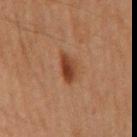The lesion was photographed on a routine skin check and not biopsied; there is no pathology result. Approximately 3.5 mm at its widest. The lesion-visualizer software estimated border irregularity of about 2.5 on a 0–10 scale, internal color variation of about 4 on a 0–10 scale, and radial color variation of about 1. And it measured a nevus-likeness score of about 95/100 and a detector confidence of about 100 out of 100 that the crop contains a lesion. The lesion is on the mid back. A 15 mm close-up tile from a total-body photography series done for melanoma screening. A male subject, aged 63 to 67.